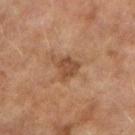No biopsy was performed on this lesion — it was imaged during a full skin examination and was not determined to be concerning.